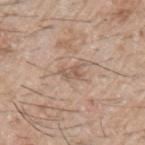The lesion was tiled from a total-body skin photograph and was not biopsied.
Located on the arm.
Captured under white-light illumination.
The recorded lesion diameter is about 2.5 mm.
A 15 mm close-up tile from a total-body photography series done for melanoma screening.
The patient is a male approximately 65 years of age.
An algorithmic analysis of the crop reported a lesion area of about 3 mm². And it measured a lesion–skin lightness drop of about 8 and a normalized lesion–skin contrast near 6.